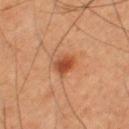notes: total-body-photography surveillance lesion; no biopsy | imaging modality: ~15 mm tile from a whole-body skin photo | illumination: cross-polarized illumination | anatomic site: the right thigh | automated metrics: a mean CIELAB color near L≈44 a*≈26 b*≈34, a lesion–skin lightness drop of about 11, and a lesion-to-skin contrast of about 9 (normalized; higher = more distinct); a border-irregularity rating of about 2/10, internal color variation of about 3.5 on a 0–10 scale, and peripheral color asymmetry of about 1.5; a classifier nevus-likeness of about 95/100 | subject: male, in their 60s | lesion size: ~2.5 mm (longest diameter).